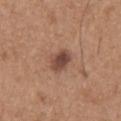No biopsy was performed on this lesion — it was imaged during a full skin examination and was not determined to be concerning.
The subject is a male aged 63–67.
From the chest.
The tile uses white-light illumination.
Measured at roughly 3 mm in maximum diameter.
A 15 mm crop from a total-body photograph taken for skin-cancer surveillance.